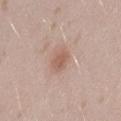biopsy_status: not biopsied; imaged during a skin examination
site: left thigh
patient:
  sex: female
  age_approx: 25
image:
  source: total-body photography crop
  field_of_view_mm: 15
lighting: white-light
lesion_size:
  long_diameter_mm_approx: 2.5
automated_metrics:
  area_mm2_approx: 4.0
  eccentricity: 0.65
  shape_asymmetry: 0.2
  lesion_detection_confidence_0_100: 100